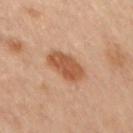Impression:
The lesion was tiled from a total-body skin photograph and was not biopsied.
Image and clinical context:
A close-up tile cropped from a whole-body skin photograph, about 15 mm across. An algorithmic analysis of the crop reported a lesion area of about 10 mm² and a shape-asymmetry score of about 0.15 (0 = symmetric). And it measured a mean CIELAB color near L≈46 a*≈21 b*≈31, about 11 CIELAB-L* units darker than the surrounding skin, and a lesion-to-skin contrast of about 8.5 (normalized; higher = more distinct). And it measured a border-irregularity rating of about 1.5/10, a within-lesion color-variation index near 2.5/10, and peripheral color asymmetry of about 1. The analysis additionally found a nevus-likeness score of about 95/100. The lesion is on the left arm. Imaged with cross-polarized lighting. The patient is a female aged 58 to 62.